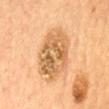The lesion was photographed on a routine skin check and not biopsied; there is no pathology result. A female subject in their 60s. The lesion is on the front of the torso. The lesion-visualizer software estimated an outline eccentricity of about 0.75 (0 = round, 1 = elongated) and two-axis asymmetry of about 0.15. The analysis additionally found a border-irregularity rating of about 2/10, internal color variation of about 6.5 on a 0–10 scale, and radial color variation of about 2. And it measured an automated nevus-likeness rating near 0 out of 100 and lesion-presence confidence of about 100/100. This image is a 15 mm lesion crop taken from a total-body photograph.